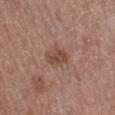Notes:
- follow-up: total-body-photography surveillance lesion; no biopsy
- size: ≈3 mm
- subject: female, aged approximately 50
- image source: 15 mm crop, total-body photography
- illumination: white-light illumination
- location: the left lower leg